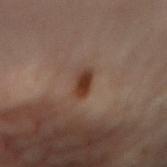This lesion was catalogued during total-body skin photography and was not selected for biopsy.
Located on the chest.
Captured under cross-polarized illumination.
A female patient, approximately 50 years of age.
Cropped from a whole-body photographic skin survey; the tile spans about 15 mm.
About 3 mm across.